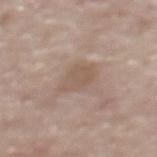This lesion was catalogued during total-body skin photography and was not selected for biopsy.
The subject is a male aged around 85 or older.
Longest diameter approximately 3.5 mm.
The total-body-photography lesion software estimated a lesion-detection confidence of about 100/100.
The tile uses white-light illumination.
On the upper back.
A roughly 15 mm field-of-view crop from a total-body skin photograph.